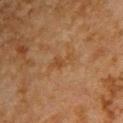No biopsy was performed on this lesion — it was imaged during a full skin examination and was not determined to be concerning. The lesion is on the upper back. A 15 mm close-up tile from a total-body photography series done for melanoma screening. Automated image analysis of the tile measured an average lesion color of about L≈37 a*≈17 b*≈30 (CIELAB) and a normalized lesion–skin contrast near 5.5. The software also gave border irregularity of about 4.5 on a 0–10 scale, a color-variation rating of about 1.5/10, and a peripheral color-asymmetry measure near 0.5. And it measured a classifier nevus-likeness of about 0/100 and lesion-presence confidence of about 100/100. Longest diameter approximately 3 mm. A male patient in their mid- to late 60s.